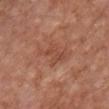biopsy_status: not biopsied; imaged during a skin examination
lighting: white-light
automated_metrics:
  eccentricity: 0.8
  shape_asymmetry: 0.4
  cielab_L: 48
  cielab_a: 24
  cielab_b: 30
  vs_skin_darker_L: 7.0
  vs_skin_contrast_norm: 5.0
  border_irregularity_0_10: 5.5
  color_variation_0_10: 2.5
  peripheral_color_asymmetry: 1.0
  nevus_likeness_0_100: 0
  lesion_detection_confidence_0_100: 100
site: chest
lesion_size:
  long_diameter_mm_approx: 4.0
image:
  source: total-body photography crop
  field_of_view_mm: 15
patient:
  sex: male
  age_approx: 65